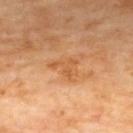Assessment:
Recorded during total-body skin imaging; not selected for excision or biopsy.
Image and clinical context:
Measured at roughly 3.5 mm in maximum diameter. The patient is a male in their 70s. The lesion is on the upper back. A 15 mm close-up tile from a total-body photography series done for melanoma screening.